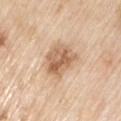biopsy_status: not biopsied; imaged during a skin examination
site: arm
image:
  source: total-body photography crop
  field_of_view_mm: 15
patient:
  sex: male
  age_approx: 80